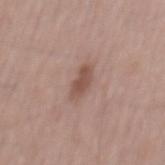Imaged during a routine full-body skin examination; the lesion was not biopsied and no histopathology is available. The subject is a male aged 53–57. A region of skin cropped from a whole-body photographic capture, roughly 15 mm wide.